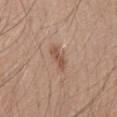Part of a total-body skin-imaging series; this lesion was reviewed on a skin check and was not flagged for biopsy.
The subject is a male about 40 years old.
This image is a 15 mm lesion crop taken from a total-body photograph.
From the mid back.